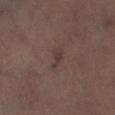Part of a total-body skin-imaging series; this lesion was reviewed on a skin check and was not flagged for biopsy.
A close-up tile cropped from a whole-body skin photograph, about 15 mm across.
About 3 mm across.
On the left lower leg.
The total-body-photography lesion software estimated a border-irregularity index near 4/10, a within-lesion color-variation index near 1/10, and peripheral color asymmetry of about 0. The analysis additionally found an automated nevus-likeness rating near 0 out of 100 and lesion-presence confidence of about 95/100.
This is a cross-polarized tile.